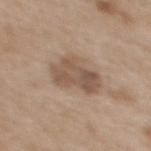The lesion was photographed on a routine skin check and not biopsied; there is no pathology result. Longest diameter approximately 5 mm. The tile uses white-light illumination. The subject is a female aged 63–67. From the upper back. A 15 mm crop from a total-body photograph taken for skin-cancer surveillance.